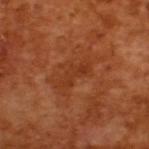Part of a total-body skin-imaging series; this lesion was reviewed on a skin check and was not flagged for biopsy.